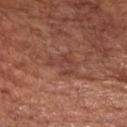Clinical summary: The lesion is located on the chest. The subject is a male roughly 65 years of age. Cropped from a whole-body photographic skin survey; the tile spans about 15 mm. Automated tile analysis of the lesion measured a lesion-to-skin contrast of about 5.5 (normalized; higher = more distinct). Captured under white-light illumination.